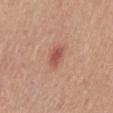{"biopsy_status": "not biopsied; imaged during a skin examination", "image": {"source": "total-body photography crop", "field_of_view_mm": 15}, "lighting": "white-light", "site": "mid back", "lesion_size": {"long_diameter_mm_approx": 2.5}, "patient": {"sex": "male", "age_approx": 55}}